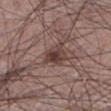Captured during whole-body skin photography for melanoma surveillance; the lesion was not biopsied. About 4 mm across. Imaged with white-light lighting. A male subject roughly 60 years of age. A 15 mm close-up tile from a total-body photography series done for melanoma screening. Located on the left lower leg.